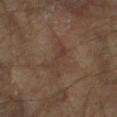Impression: Part of a total-body skin-imaging series; this lesion was reviewed on a skin check and was not flagged for biopsy. Background: Captured under cross-polarized illumination. A lesion tile, about 15 mm wide, cut from a 3D total-body photograph. A male patient about 65 years old. From the right forearm.